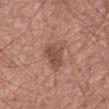Background: Located on the mid back. A male subject in their mid-50s. Cropped from a whole-body photographic skin survey; the tile spans about 15 mm.